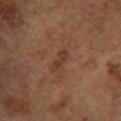biopsy status: total-body-photography surveillance lesion; no biopsy | image: 15 mm crop, total-body photography | body site: the leg | illumination: cross-polarized | patient: female, roughly 65 years of age.